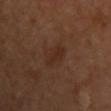Case summary:
• biopsy status: catalogued during a skin exam; not biopsied
• size: about 3.5 mm
• TBP lesion metrics: a mean CIELAB color near L≈28 a*≈19 b*≈26, roughly 5 lightness units darker than nearby skin, and a normalized lesion–skin contrast near 6; a classifier nevus-likeness of about 5/100 and a detector confidence of about 100 out of 100 that the crop contains a lesion
• acquisition: ~15 mm crop, total-body skin-cancer survey
• location: the chest
• subject: female, aged 38 to 42